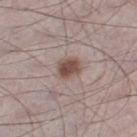Q: Is there a histopathology result?
A: catalogued during a skin exam; not biopsied
Q: How was this image acquired?
A: ~15 mm crop, total-body skin-cancer survey
Q: Where on the body is the lesion?
A: the left thigh
Q: How was the tile lit?
A: white-light
Q: How large is the lesion?
A: ≈3 mm
Q: Automated lesion metrics?
A: a border-irregularity index near 1.5/10 and peripheral color asymmetry of about 1
Q: What are the patient's age and sex?
A: male, about 70 years old